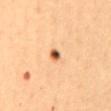Part of a total-body skin-imaging series; this lesion was reviewed on a skin check and was not flagged for biopsy.
From the mid back.
The lesion-visualizer software estimated border irregularity of about 2 on a 0–10 scale, internal color variation of about 5.5 on a 0–10 scale, and a peripheral color-asymmetry measure near 2. It also reported an automated nevus-likeness rating near 100 out of 100 and a lesion-detection confidence of about 100/100.
A female patient in their mid- to late 60s.
Measured at roughly 1.5 mm in maximum diameter.
A 15 mm close-up tile from a total-body photography series done for melanoma screening.
The tile uses cross-polarized illumination.